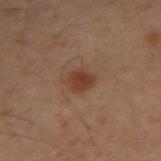Q: Illumination type?
A: cross-polarized
Q: How large is the lesion?
A: ~2.5 mm (longest diameter)
Q: What kind of image is this?
A: total-body-photography crop, ~15 mm field of view
Q: What are the patient's age and sex?
A: male, aged around 50
Q: What did automated image analysis measure?
A: a mean CIELAB color near L≈31 a*≈17 b*≈25, about 7 CIELAB-L* units darker than the surrounding skin, and a normalized border contrast of about 8.5; a nevus-likeness score of about 85/100 and a lesion-detection confidence of about 100/100
Q: What is the anatomic site?
A: the left arm